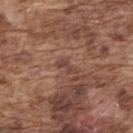This lesion was catalogued during total-body skin photography and was not selected for biopsy. A lesion tile, about 15 mm wide, cut from a 3D total-body photograph. Captured under white-light illumination. The lesion is on the upper back. The lesion's longest dimension is about 2.5 mm. A male subject, approximately 75 years of age. The lesion-visualizer software estimated a lesion area of about 2 mm², a shape eccentricity near 0.9, and a shape-asymmetry score of about 0.55 (0 = symmetric). The software also gave border irregularity of about 6.5 on a 0–10 scale. The analysis additionally found lesion-presence confidence of about 95/100.